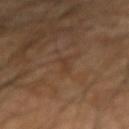Cropped from a total-body skin-imaging series; the visible field is about 15 mm.
A male subject aged approximately 70.
From the arm.